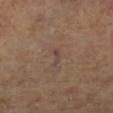| field | value |
|---|---|
| follow-up | no biopsy performed (imaged during a skin exam) |
| imaging modality | ~15 mm crop, total-body skin-cancer survey |
| patient | female, aged 68–72 |
| size | ~3 mm (longest diameter) |
| body site | the left lower leg |
| lighting | cross-polarized |